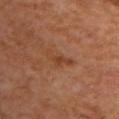Imaged during a routine full-body skin examination; the lesion was not biopsied and no histopathology is available.
On the back.
An algorithmic analysis of the crop reported border irregularity of about 6 on a 0–10 scale, internal color variation of about 1 on a 0–10 scale, and a peripheral color-asymmetry measure near 0.5.
Cropped from a whole-body photographic skin survey; the tile spans about 15 mm.
A female subject.
The tile uses cross-polarized illumination.
About 3.5 mm across.